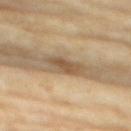biopsy status: no biopsy performed (imaged during a skin exam)
lighting: cross-polarized illumination
patient: female, approximately 75 years of age
site: the chest
acquisition: 15 mm crop, total-body photography
image-analysis metrics: a lesion area of about 6 mm², a shape eccentricity near 0.8, and a shape-asymmetry score of about 0.25 (0 = symmetric); a mean CIELAB color near L≈53 a*≈14 b*≈33, roughly 11 lightness units darker than nearby skin, and a normalized lesion–skin contrast near 7.5; a detector confidence of about 55 out of 100 that the crop contains a lesion